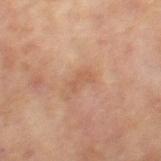follow-up: catalogued during a skin exam; not biopsied | automated metrics: a shape eccentricity near 0.9 and two-axis asymmetry of about 0.4; roughly 6 lightness units darker than nearby skin and a lesion-to-skin contrast of about 4.5 (normalized; higher = more distinct); a border-irregularity index near 4.5/10, internal color variation of about 0 on a 0–10 scale, and a peripheral color-asymmetry measure near 0 | lesion diameter: ≈2.5 mm | patient: female, in their 60s | lighting: cross-polarized | acquisition: ~15 mm crop, total-body skin-cancer survey | location: the leg.